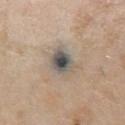Impression: The lesion was photographed on a routine skin check and not biopsied; there is no pathology result. Background: Cropped from a whole-body photographic skin survey; the tile spans about 15 mm. The recorded lesion diameter is about 3.5 mm. Imaged with white-light lighting. The lesion is located on the chest. A female subject, in their mid- to late 40s. Automated image analysis of the tile measured a lesion color around L≈52 a*≈6 b*≈17 in CIELAB, about 14 CIELAB-L* units darker than the surrounding skin, and a normalized lesion–skin contrast near 12. The software also gave a border-irregularity rating of about 2/10 and internal color variation of about 7.5 on a 0–10 scale. The analysis additionally found an automated nevus-likeness rating near 20 out of 100 and a lesion-detection confidence of about 60/100.